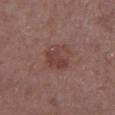• follow-up — catalogued during a skin exam; not biopsied
• subject — male, aged around 70
• lesion diameter — ~4 mm (longest diameter)
• body site — the left lower leg
• TBP lesion metrics — a border-irregularity rating of about 3.5/10 and a within-lesion color-variation index near 2.5/10; a nevus-likeness score of about 15/100 and a detector confidence of about 100 out of 100 that the crop contains a lesion
• imaging modality — ~15 mm crop, total-body skin-cancer survey